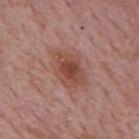lesion diameter: ≈4.5 mm
site: the back
automated lesion analysis: an area of roughly 12 mm², an eccentricity of roughly 0.75, and two-axis asymmetry of about 0.25; a mean CIELAB color near L≈47 a*≈23 b*≈26, about 9 CIELAB-L* units darker than the surrounding skin, and a normalized border contrast of about 7.5; a classifier nevus-likeness of about 90/100 and lesion-presence confidence of about 100/100
image: total-body-photography crop, ~15 mm field of view
subject: male, aged 73–77
lighting: white-light illumination